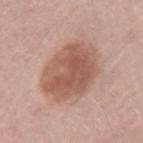This lesion was catalogued during total-body skin photography and was not selected for biopsy. A 15 mm close-up extracted from a 3D total-body photography capture. A female subject, approximately 60 years of age. The lesion is located on the left upper arm.